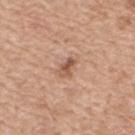- biopsy status · total-body-photography surveillance lesion; no biopsy
- TBP lesion metrics · a lesion area of about 3.5 mm², a shape eccentricity near 0.8, and two-axis asymmetry of about 0.3; a mean CIELAB color near L≈55 a*≈22 b*≈31 and roughly 11 lightness units darker than nearby skin
- tile lighting · white-light
- image · total-body-photography crop, ~15 mm field of view
- size · ~2.5 mm (longest diameter)
- patient · female, about 55 years old
- location · the upper back